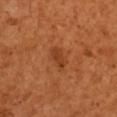site: the arm
diameter: ~2.5 mm (longest diameter)
imaging modality: ~15 mm tile from a whole-body skin photo
subject: male, approximately 50 years of age
automated lesion analysis: a footprint of about 3.5 mm², an eccentricity of roughly 0.85, and a shape-asymmetry score of about 0.25 (0 = symmetric); border irregularity of about 2.5 on a 0–10 scale and radial color variation of about 0.5; an automated nevus-likeness rating near 55 out of 100 and a lesion-detection confidence of about 100/100